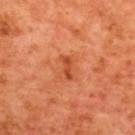follow-up: catalogued during a skin exam; not biopsied
TBP lesion metrics: an area of roughly 3 mm² and an eccentricity of roughly 0.9; a lesion color around L≈49 a*≈34 b*≈41 in CIELAB and a lesion–skin lightness drop of about 9
location: the upper back
lesion size: ~2.5 mm (longest diameter)
image source: total-body-photography crop, ~15 mm field of view
patient: male, aged 63–67